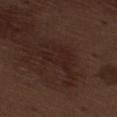Imaged during a routine full-body skin examination; the lesion was not biopsied and no histopathology is available.
This is a white-light tile.
Measured at roughly 6.5 mm in maximum diameter.
The lesion is on the right thigh.
A male subject, about 70 years old.
A 15 mm close-up tile from a total-body photography series done for melanoma screening.